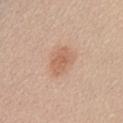A female subject, approximately 45 years of age.
The lesion is located on the abdomen.
Automated tile analysis of the lesion measured an area of roughly 7.5 mm² and a symmetry-axis asymmetry near 0.25. It also reported a border-irregularity rating of about 2.5/10, internal color variation of about 2 on a 0–10 scale, and radial color variation of about 0.5.
About 3.5 mm across.
Cropped from a whole-body photographic skin survey; the tile spans about 15 mm.